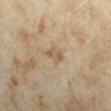Background:
On the left forearm. Cropped from a total-body skin-imaging series; the visible field is about 15 mm. A female patient, aged 33–37.